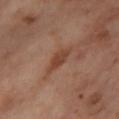Clinical summary:
The recorded lesion diameter is about 3.5 mm. The lesion is located on the right thigh. The tile uses cross-polarized illumination. A female subject, about 55 years old. A 15 mm crop from a total-body photograph taken for skin-cancer surveillance.